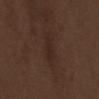Acquisition and patient details: Automated image analysis of the tile measured an area of roughly 5.5 mm², an outline eccentricity of about 0.95 (0 = round, 1 = elongated), and a shape-asymmetry score of about 0.25 (0 = symmetric). It also reported border irregularity of about 3.5 on a 0–10 scale, internal color variation of about 1.5 on a 0–10 scale, and a peripheral color-asymmetry measure near 0.5. A male subject, aged 68–72. Imaged with white-light lighting. Cropped from a whole-body photographic skin survey; the tile spans about 15 mm. On the front of the torso.